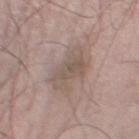Captured during whole-body skin photography for melanoma surveillance; the lesion was not biopsied. The tile uses white-light illumination. Cropped from a whole-body photographic skin survey; the tile spans about 15 mm. On the left lower leg. The subject is a male aged around 50. Approximately 6 mm at its widest.